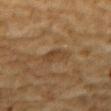Context: Measured at roughly 3.5 mm in maximum diameter. The lesion is on the mid back. This image is a 15 mm lesion crop taken from a total-body photograph. The subject is a male approximately 85 years of age or older.